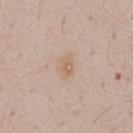- biopsy status: imaged on a skin check; not biopsied
- lesion size: ~2.5 mm (longest diameter)
- location: the chest
- subject: male, aged 48 to 52
- imaging modality: ~15 mm crop, total-body skin-cancer survey
- lighting: white-light